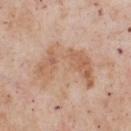lesion diameter=about 7 mm
automated lesion analysis=border irregularity of about 9.5 on a 0–10 scale, internal color variation of about 4 on a 0–10 scale, and peripheral color asymmetry of about 1.5; an automated nevus-likeness rating near 5 out of 100 and lesion-presence confidence of about 100/100
illumination=white-light
image=total-body-photography crop, ~15 mm field of view
body site=the front of the torso
patient=male, approximately 55 years of age